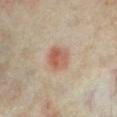{"biopsy_status": "not biopsied; imaged during a skin examination", "site": "left lower leg", "lesion_size": {"long_diameter_mm_approx": 3.0}, "patient": {"sex": "female", "age_approx": 35}, "image": {"source": "total-body photography crop", "field_of_view_mm": 15}}